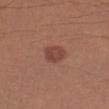- image-analysis metrics: a lesion area of about 6 mm², an eccentricity of roughly 0.25, and two-axis asymmetry of about 0.2; a mean CIELAB color near L≈44 a*≈23 b*≈27, about 9 CIELAB-L* units darker than the surrounding skin, and a lesion-to-skin contrast of about 7 (normalized; higher = more distinct)
- patient: male, aged approximately 35
- body site: the left forearm
- image: 15 mm crop, total-body photography
- tile lighting: white-light illumination